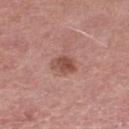  biopsy_status: not biopsied; imaged during a skin examination
  automated_metrics:
    eccentricity: 0.7
    shape_asymmetry: 0.25
    color_variation_0_10: 4.0
    peripheral_color_asymmetry: 1.5
  site: leg
  image:
    source: total-body photography crop
    field_of_view_mm: 15
  lesion_size:
    long_diameter_mm_approx: 3.0
  lighting: white-light
  patient:
    sex: female
    age_approx: 70